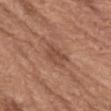From the abdomen. The patient is a female aged 63–67. A roughly 15 mm field-of-view crop from a total-body skin photograph. Automated image analysis of the tile measured roughly 8 lightness units darker than nearby skin. And it measured a border-irregularity index near 2.5/10, a within-lesion color-variation index near 2.5/10, and peripheral color asymmetry of about 1. It also reported a detector confidence of about 100 out of 100 that the crop contains a lesion. Longest diameter approximately 3 mm.